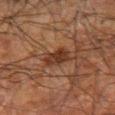<lesion>
<biopsy_status>not biopsied; imaged during a skin examination</biopsy_status>
<patient>
  <sex>male</sex>
  <age_approx>70</age_approx>
</patient>
<image>
  <source>total-body photography crop</source>
  <field_of_view_mm>15</field_of_view_mm>
</image>
<site>right forearm</site>
</lesion>